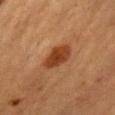notes: catalogued during a skin exam; not biopsied
acquisition: total-body-photography crop, ~15 mm field of view
lesion size: ~4.5 mm (longest diameter)
body site: the chest
tile lighting: cross-polarized
patient: female, aged 38 to 42